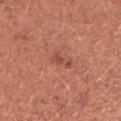Part of a total-body skin-imaging series; this lesion was reviewed on a skin check and was not flagged for biopsy. The tile uses white-light illumination. A 15 mm close-up extracted from a 3D total-body photography capture. On the arm. The patient is a female in their mid- to late 30s.